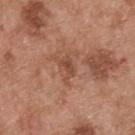Clinical impression: Recorded during total-body skin imaging; not selected for excision or biopsy. Clinical summary: The lesion is on the upper back. A male patient aged 53 to 57. This is a white-light tile. A close-up tile cropped from a whole-body skin photograph, about 15 mm across.